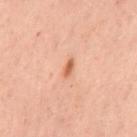Impression:
No biopsy was performed on this lesion — it was imaged during a full skin examination and was not determined to be concerning.
Clinical summary:
An algorithmic analysis of the crop reported an area of roughly 2.5 mm², an eccentricity of roughly 0.9, and a shape-asymmetry score of about 0.4 (0 = symmetric). Cropped from a whole-body photographic skin survey; the tile spans about 15 mm. The lesion is located on the mid back. The patient is a female aged around 55.